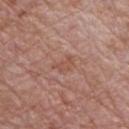patient = male, about 70 years old | acquisition = total-body-photography crop, ~15 mm field of view | site = the front of the torso.